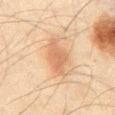notes = total-body-photography surveillance lesion; no biopsy
patient = male, aged 43 to 47
location = the abdomen
acquisition = total-body-photography crop, ~15 mm field of view